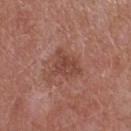This lesion was catalogued during total-body skin photography and was not selected for biopsy.
The lesion is on the upper back.
The patient is a female roughly 60 years of age.
Cropped from a whole-body photographic skin survey; the tile spans about 15 mm.
This is a white-light tile.
About 4.5 mm across.